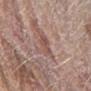Impression: The lesion was tiled from a total-body skin photograph and was not biopsied. Background: A male subject, aged 78 to 82. A 15 mm close-up tile from a total-body photography series done for melanoma screening. Measured at roughly 3 mm in maximum diameter. This is a white-light tile. Located on the right forearm.